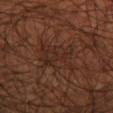Assessment: This lesion was catalogued during total-body skin photography and was not selected for biopsy. Image and clinical context: A 15 mm close-up tile from a total-body photography series done for melanoma screening. The subject is a male in their mid-50s. From the left lower leg.